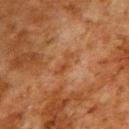- biopsy status: catalogued during a skin exam; not biopsied
- patient: male, about 80 years old
- image source: ~15 mm tile from a whole-body skin photo
- illumination: cross-polarized
- size: ~3 mm (longest diameter)
- body site: the upper back